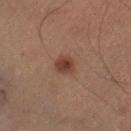Q: Was this lesion biopsied?
A: catalogued during a skin exam; not biopsied